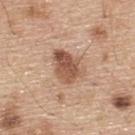Q: Is there a histopathology result?
A: total-body-photography surveillance lesion; no biopsy
Q: Lesion location?
A: the upper back
Q: Illumination type?
A: white-light
Q: How was this image acquired?
A: total-body-photography crop, ~15 mm field of view
Q: Lesion size?
A: ≈4 mm
Q: What did automated image analysis measure?
A: border irregularity of about 3.5 on a 0–10 scale, a color-variation rating of about 5.5/10, and a peripheral color-asymmetry measure near 2; an automated nevus-likeness rating near 80 out of 100 and a detector confidence of about 100 out of 100 that the crop contains a lesion
Q: Patient demographics?
A: male, roughly 70 years of age